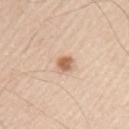follow-up: catalogued during a skin exam; not biopsied
image: ~15 mm tile from a whole-body skin photo
location: the back
subject: male, about 45 years old
diameter: ≈2.5 mm
TBP lesion metrics: a lesion area of about 4 mm², an eccentricity of roughly 0.7, and two-axis asymmetry of about 0.25; a border-irregularity index near 2/10, a color-variation rating of about 3.5/10, and a peripheral color-asymmetry measure near 1; a lesion-detection confidence of about 100/100